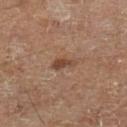Impression:
This lesion was catalogued during total-body skin photography and was not selected for biopsy.
Image and clinical context:
The subject is in their mid-50s. Imaged with cross-polarized lighting. Automated image analysis of the tile measured an eccentricity of roughly 0.85 and two-axis asymmetry of about 0.3. It also reported a border-irregularity index near 3/10, internal color variation of about 1 on a 0–10 scale, and a peripheral color-asymmetry measure near 0.5. A 15 mm crop from a total-body photograph taken for skin-cancer surveillance. Located on the left lower leg. The recorded lesion diameter is about 3 mm.